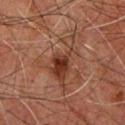This lesion was catalogued during total-body skin photography and was not selected for biopsy. Cropped from a whole-body photographic skin survey; the tile spans about 15 mm. Measured at roughly 7 mm in maximum diameter. Imaged with cross-polarized lighting. On the upper back. A male subject, aged around 60.